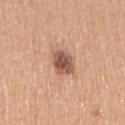Part of a total-body skin-imaging series; this lesion was reviewed on a skin check and was not flagged for biopsy. Cropped from a whole-body photographic skin survey; the tile spans about 15 mm. A female patient, aged 63 to 67. On the right upper arm.